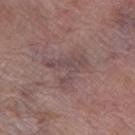Q: Was a biopsy performed?
A: imaged on a skin check; not biopsied
Q: What are the patient's age and sex?
A: female, about 70 years old
Q: What is the lesion's diameter?
A: ~5 mm (longest diameter)
Q: What lighting was used for the tile?
A: white-light illumination
Q: How was this image acquired?
A: ~15 mm tile from a whole-body skin photo
Q: Where on the body is the lesion?
A: the left thigh